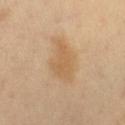Findings:
– workup: imaged on a skin check; not biopsied
– body site: the right thigh
– image: ~15 mm crop, total-body skin-cancer survey
– subject: male, aged approximately 40